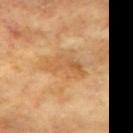notes: total-body-photography surveillance lesion; no biopsy
acquisition: 15 mm crop, total-body photography
patient: female, about 75 years old
lesion diameter: about 5 mm
image-analysis metrics: roughly 8 lightness units darker than nearby skin and a lesion-to-skin contrast of about 5 (normalized; higher = more distinct); a color-variation rating of about 5/10; a classifier nevus-likeness of about 0/100 and lesion-presence confidence of about 95/100
anatomic site: the left upper arm